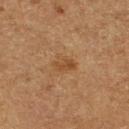Findings:
* body site · the right lower leg
* TBP lesion metrics · a lesion area of about 3.5 mm², an outline eccentricity of about 0.8 (0 = round, 1 = elongated), and a shape-asymmetry score of about 0.4 (0 = symmetric); a border-irregularity index near 4/10, a within-lesion color-variation index near 1/10, and radial color variation of about 0.5
* imaging modality · ~15 mm tile from a whole-body skin photo
* lighting · cross-polarized illumination
* patient · male, in their mid- to late 70s
* size · ~2.5 mm (longest diameter)